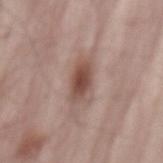This lesion was catalogued during total-body skin photography and was not selected for biopsy.
The recorded lesion diameter is about 4.5 mm.
Automated image analysis of the tile measured a footprint of about 7 mm² and a shape eccentricity near 0.9. The software also gave a lesion color around L≈48 a*≈19 b*≈24 in CIELAB, about 13 CIELAB-L* units darker than the surrounding skin, and a normalized border contrast of about 9.5. The analysis additionally found border irregularity of about 3 on a 0–10 scale, internal color variation of about 4.5 on a 0–10 scale, and radial color variation of about 1.5.
A male patient in their mid-60s.
A 15 mm crop from a total-body photograph taken for skin-cancer surveillance.
Located on the mid back.